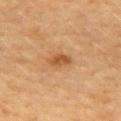| feature | finding |
|---|---|
| workup | no biopsy performed (imaged during a skin exam) |
| acquisition | ~15 mm crop, total-body skin-cancer survey |
| location | the upper back |
| patient | female, about 60 years old |